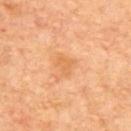The lesion was photographed on a routine skin check and not biopsied; there is no pathology result. This image is a 15 mm lesion crop taken from a total-body photograph. A female patient, in their mid- to late 60s. About 2.5 mm across. From the back.